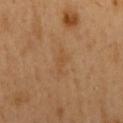workup: no biopsy performed (imaged during a skin exam)
tile lighting: cross-polarized illumination
automated lesion analysis: an eccentricity of roughly 0.9 and a shape-asymmetry score of about 0.3 (0 = symmetric); a lesion color around L≈49 a*≈19 b*≈37 in CIELAB, about 5 CIELAB-L* units darker than the surrounding skin, and a normalized lesion–skin contrast near 4.5
patient: male, aged 48–52
image: ~15 mm crop, total-body skin-cancer survey
body site: the mid back
lesion diameter: about 3 mm